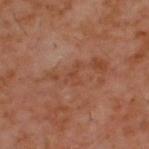Clinical impression: Captured during whole-body skin photography for melanoma surveillance; the lesion was not biopsied. Clinical summary: The patient is a male aged 58 to 62. A close-up tile cropped from a whole-body skin photograph, about 15 mm across. The lesion is on the upper back.